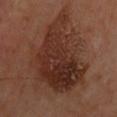Captured during whole-body skin photography for melanoma surveillance; the lesion was not biopsied. A male subject, aged around 65. Approximately 10 mm at its widest. A lesion tile, about 15 mm wide, cut from a 3D total-body photograph. The lesion is on the upper back. Imaged with cross-polarized lighting.